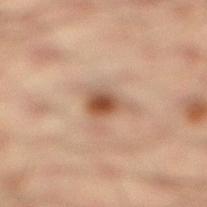follow-up = total-body-photography surveillance lesion; no biopsy
body site = the right lower leg
image source = total-body-photography crop, ~15 mm field of view
illumination = cross-polarized illumination
TBP lesion metrics = an automated nevus-likeness rating near 95 out of 100 and a detector confidence of about 100 out of 100 that the crop contains a lesion
patient = male, roughly 35 years of age